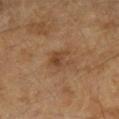  biopsy_status: not biopsied; imaged during a skin examination
  image:
    source: total-body photography crop
    field_of_view_mm: 15
  patient:
    sex: female
    age_approx: 60
  site: left forearm
  lesion_size:
    long_diameter_mm_approx: 3.0